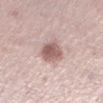{"site": "left lower leg", "image": {"source": "total-body photography crop", "field_of_view_mm": 15}, "lesion_size": {"long_diameter_mm_approx": 4.0}, "patient": {"sex": "female", "age_approx": 40}, "automated_metrics": {"area_mm2_approx": 8.5, "eccentricity": 0.7, "shape_asymmetry": 0.15, "border_irregularity_0_10": 2.0, "color_variation_0_10": 3.5, "nevus_likeness_0_100": 80, "lesion_detection_confidence_0_100": 100}, "lighting": "white-light"}